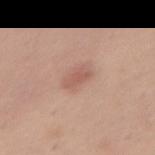imaging modality: 15 mm crop, total-body photography
TBP lesion metrics: an area of roughly 5 mm², an eccentricity of roughly 0.85, and a shape-asymmetry score of about 0.2 (0 = symmetric)
location: the abdomen
lesion size: about 3.5 mm
tile lighting: white-light illumination
patient: female, aged approximately 55